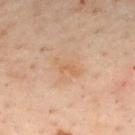{"lesion_size": {"long_diameter_mm_approx": 3.5}, "automated_metrics": {"area_mm2_approx": 3.5, "shape_asymmetry": 0.4, "border_irregularity_0_10": 4.5, "color_variation_0_10": 0.0}, "lighting": "cross-polarized", "site": "back", "patient": {"sex": "male", "age_approx": 50}, "image": {"source": "total-body photography crop", "field_of_view_mm": 15}}